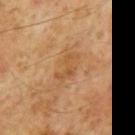Imaged during a routine full-body skin examination; the lesion was not biopsied and no histopathology is available. The tile uses cross-polarized illumination. Cropped from a total-body skin-imaging series; the visible field is about 15 mm. A male subject about 60 years old. On the mid back.